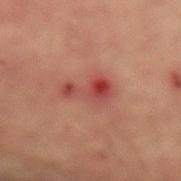Q: Was a biopsy performed?
A: total-body-photography surveillance lesion; no biopsy
Q: What is the anatomic site?
A: the lower back
Q: What are the patient's age and sex?
A: male, in their mid- to late 60s
Q: How large is the lesion?
A: ≈4.5 mm
Q: How was this image acquired?
A: total-body-photography crop, ~15 mm field of view